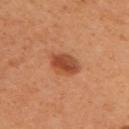* follow-up: total-body-photography surveillance lesion; no biopsy
* lesion size: ≈4 mm
* acquisition: ~15 mm crop, total-body skin-cancer survey
* anatomic site: the upper back
* patient: female, aged approximately 40
* image-analysis metrics: an automated nevus-likeness rating near 95 out of 100 and lesion-presence confidence of about 100/100
* tile lighting: cross-polarized